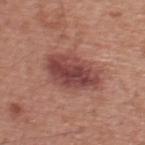<lesion>
<biopsy_status>not biopsied; imaged during a skin examination</biopsy_status>
<image>
  <source>total-body photography crop</source>
  <field_of_view_mm>15</field_of_view_mm>
</image>
<patient>
  <sex>male</sex>
  <age_approx>60</age_approx>
</patient>
<site>upper back</site>
</lesion>